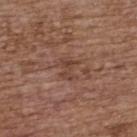Cropped from a total-body skin-imaging series; the visible field is about 15 mm. The lesion-visualizer software estimated an eccentricity of roughly 0.7 and two-axis asymmetry of about 0.4. It also reported an average lesion color of about L≈42 a*≈20 b*≈26 (CIELAB), about 7 CIELAB-L* units darker than the surrounding skin, and a normalized border contrast of about 6. And it measured a border-irregularity rating of about 5.5/10, internal color variation of about 1 on a 0–10 scale, and radial color variation of about 0. It also reported a nevus-likeness score of about 0/100 and a lesion-detection confidence of about 85/100. A female subject, aged 63–67. The tile uses white-light illumination. About 2.5 mm across. The lesion is located on the upper back.